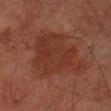This lesion was catalogued during total-body skin photography and was not selected for biopsy. An algorithmic analysis of the crop reported a mean CIELAB color near L≈34 a*≈25 b*≈28 and a normalized border contrast of about 6.5. It also reported a border-irregularity index near 5/10, a within-lesion color-variation index near 3/10, and peripheral color asymmetry of about 1. The lesion is on the left arm. Imaged with cross-polarized lighting. A male patient aged 48–52. A region of skin cropped from a whole-body photographic capture, roughly 15 mm wide. Longest diameter approximately 8 mm.